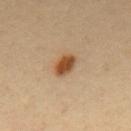The lesion was tiled from a total-body skin photograph and was not biopsied.
The patient is a male aged around 40.
Located on the mid back.
A lesion tile, about 15 mm wide, cut from a 3D total-body photograph.